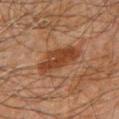Impression: The lesion was tiled from a total-body skin photograph and was not biopsied. Context: The total-body-photography lesion software estimated a mean CIELAB color near L≈32 a*≈19 b*≈27, roughly 8 lightness units darker than nearby skin, and a normalized lesion–skin contrast near 8. The analysis additionally found a border-irregularity index near 3.5/10 and a color-variation rating of about 3/10. The software also gave a classifier nevus-likeness of about 50/100 and a lesion-detection confidence of about 100/100. This image is a 15 mm lesion crop taken from a total-body photograph. The patient is a male aged 58 to 62. Approximately 6 mm at its widest. Captured under cross-polarized illumination. On the chest.